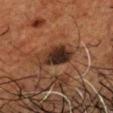Imaged during a routine full-body skin examination; the lesion was not biopsied and no histopathology is available.
A roughly 15 mm field-of-view crop from a total-body skin photograph.
From the head or neck.
Imaged with cross-polarized lighting.
The total-body-photography lesion software estimated an area of roughly 10 mm² and a shape-asymmetry score of about 0.3 (0 = symmetric). And it measured an automated nevus-likeness rating near 35 out of 100 and a detector confidence of about 100 out of 100 that the crop contains a lesion.
The patient is a male aged around 60.